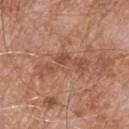Captured during whole-body skin photography for melanoma surveillance; the lesion was not biopsied. The lesion is on the upper back. This image is a 15 mm lesion crop taken from a total-body photograph. Longest diameter approximately 6 mm. A male subject, roughly 80 years of age.